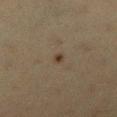Assessment: No biopsy was performed on this lesion — it was imaged during a full skin examination and was not determined to be concerning. Context: A 15 mm close-up tile from a total-body photography series done for melanoma screening. Automated image analysis of the tile measured a border-irregularity index near 2/10, internal color variation of about 0 on a 0–10 scale, and radial color variation of about 0. On the leg. Approximately 1.5 mm at its widest. A female patient, roughly 40 years of age. Imaged with cross-polarized lighting.